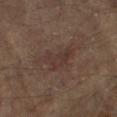Clinical impression: Captured during whole-body skin photography for melanoma surveillance; the lesion was not biopsied. Background: A male subject, aged 63 to 67. A 15 mm close-up tile from a total-body photography series done for melanoma screening. Automated tile analysis of the lesion measured a footprint of about 7 mm² and an outline eccentricity of about 0.85 (0 = round, 1 = elongated). About 4 mm across. From the right forearm. Captured under cross-polarized illumination.